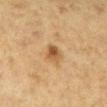Measured at roughly 3 mm in maximum diameter. From the left lower leg. Automated image analysis of the tile measured roughly 10 lightness units darker than nearby skin and a normalized border contrast of about 8. The software also gave a border-irregularity index near 2/10, internal color variation of about 5.5 on a 0–10 scale, and radial color variation of about 2. The software also gave a classifier nevus-likeness of about 70/100 and a lesion-detection confidence of about 100/100. A 15 mm crop from a total-body photograph taken for skin-cancer surveillance. Captured under cross-polarized illumination. A female subject in their mid-50s.